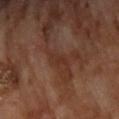Impression:
Imaged during a routine full-body skin examination; the lesion was not biopsied and no histopathology is available.
Background:
The lesion is on the back. A male subject aged 68 to 72. Cropped from a total-body skin-imaging series; the visible field is about 15 mm. About 2.5 mm across. This is a cross-polarized tile. The lesion-visualizer software estimated a footprint of about 2.5 mm², a shape eccentricity near 0.9, and a symmetry-axis asymmetry near 0.45. It also reported a lesion-detection confidence of about 50/100.